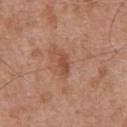No biopsy was performed on this lesion — it was imaged during a full skin examination and was not determined to be concerning. A male patient aged 73–77. Imaged with white-light lighting. A close-up tile cropped from a whole-body skin photograph, about 15 mm across. Measured at roughly 2.5 mm in maximum diameter. The lesion is on the chest.